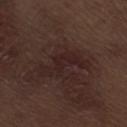<case>
  <biopsy_status>not biopsied; imaged during a skin examination</biopsy_status>
  <site>right thigh</site>
  <image>
    <source>total-body photography crop</source>
    <field_of_view_mm>15</field_of_view_mm>
  </image>
  <patient>
    <sex>male</sex>
    <age_approx>70</age_approx>
  </patient>
</case>